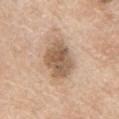Background:
Located on the chest. A male subject aged 63–67. A 15 mm close-up tile from a total-body photography series done for melanoma screening. Longest diameter approximately 5 mm.